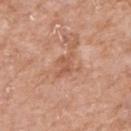Q: Is there a histopathology result?
A: no biopsy performed (imaged during a skin exam)
Q: What kind of image is this?
A: ~15 mm crop, total-body skin-cancer survey
Q: What is the anatomic site?
A: the right forearm
Q: Who is the patient?
A: female, in their mid- to late 70s
Q: How was the tile lit?
A: white-light illumination